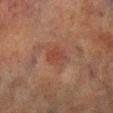Q: Was a biopsy performed?
A: catalogued during a skin exam; not biopsied
Q: How was this image acquired?
A: 15 mm crop, total-body photography
Q: What are the patient's age and sex?
A: male, roughly 70 years of age
Q: Illumination type?
A: cross-polarized
Q: Lesion size?
A: ~3 mm (longest diameter)
Q: Lesion location?
A: the left lower leg
Q: Automated lesion metrics?
A: an area of roughly 4.5 mm², a shape eccentricity near 0.7, and a shape-asymmetry score of about 0.25 (0 = symmetric); border irregularity of about 2.5 on a 0–10 scale, a within-lesion color-variation index near 2/10, and a peripheral color-asymmetry measure near 0.5; a nevus-likeness score of about 25/100 and a lesion-detection confidence of about 100/100